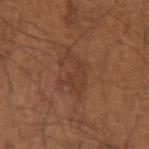biopsy status = catalogued during a skin exam; not biopsied | location = the arm | patient = male, in their mid- to late 60s | acquisition = ~15 mm tile from a whole-body skin photo | diameter = ~4.5 mm (longest diameter) | illumination = white-light illumination.